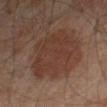Recorded during total-body skin imaging; not selected for excision or biopsy. The lesion is on the leg. The lesion's longest dimension is about 7.5 mm. Cropped from a total-body skin-imaging series; the visible field is about 15 mm. An algorithmic analysis of the crop reported a lesion area of about 29 mm², a shape eccentricity near 0.65, and a shape-asymmetry score of about 0.2 (0 = symmetric). And it measured a border-irregularity rating of about 3.5/10, a color-variation rating of about 3/10, and radial color variation of about 1. The analysis additionally found a nevus-likeness score of about 75/100 and a lesion-detection confidence of about 100/100. A male patient, roughly 60 years of age. The tile uses cross-polarized illumination.